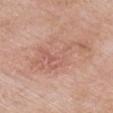{
  "biopsy_status": "not biopsied; imaged during a skin examination",
  "automated_metrics": {
    "area_mm2_approx": 15.0,
    "eccentricity": 0.95,
    "shape_asymmetry": 0.6,
    "vs_skin_darker_L": 7.0,
    "vs_skin_contrast_norm": 5.0,
    "color_variation_0_10": 3.0,
    "peripheral_color_asymmetry": 1.0
  },
  "site": "chest",
  "patient": {
    "sex": "female",
    "age_approx": 70
  },
  "image": {
    "source": "total-body photography crop",
    "field_of_view_mm": 15
  }
}